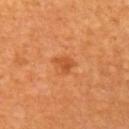Q: Was a biopsy performed?
A: catalogued during a skin exam; not biopsied
Q: Patient demographics?
A: female, aged 63 to 67
Q: What kind of image is this?
A: ~15 mm tile from a whole-body skin photo
Q: What is the lesion's diameter?
A: ≈2.5 mm
Q: What is the anatomic site?
A: the arm
Q: Illumination type?
A: cross-polarized
Q: What did automated image analysis measure?
A: a nevus-likeness score of about 60/100 and lesion-presence confidence of about 100/100